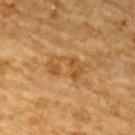workup: catalogued during a skin exam; not biopsied
patient: male, in their mid- to late 80s or older
tile lighting: cross-polarized illumination
acquisition: ~15 mm tile from a whole-body skin photo
body site: the upper back